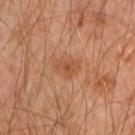Q: Was a biopsy performed?
A: imaged on a skin check; not biopsied
Q: What is the anatomic site?
A: the left forearm
Q: Automated lesion metrics?
A: a lesion color around L≈48 a*≈23 b*≈33 in CIELAB, about 7 CIELAB-L* units darker than the surrounding skin, and a normalized border contrast of about 5.5
Q: What is the lesion's diameter?
A: ≈3 mm
Q: Who is the patient?
A: male, aged approximately 45
Q: How was the tile lit?
A: cross-polarized
Q: How was this image acquired?
A: ~15 mm tile from a whole-body skin photo